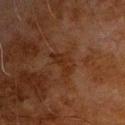Image and clinical context: The subject is a male aged around 80. Located on the chest. Longest diameter approximately 3.5 mm. This image is a 15 mm lesion crop taken from a total-body photograph. Imaged with cross-polarized lighting. Automated image analysis of the tile measured a footprint of about 5.5 mm², a shape eccentricity near 0.85, and a shape-asymmetry score of about 0.55 (0 = symmetric). The analysis additionally found a border-irregularity index near 6/10 and a peripheral color-asymmetry measure near 0.5. The software also gave a lesion-detection confidence of about 100/100.